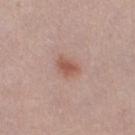- biopsy status · no biopsy performed (imaged during a skin exam)
- image-analysis metrics · a mean CIELAB color near L≈55 a*≈22 b*≈27, roughly 9 lightness units darker than nearby skin, and a normalized lesion–skin contrast near 7; a lesion-detection confidence of about 100/100
- location · the right thigh
- imaging modality · ~15 mm crop, total-body skin-cancer survey
- subject · female, aged around 40
- lesion size · ≈3 mm